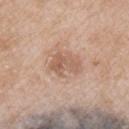Assessment: No biopsy was performed on this lesion — it was imaged during a full skin examination and was not determined to be concerning. Context: Imaged with white-light lighting. On the right upper arm. The patient is a male about 65 years old. A 15 mm close-up extracted from a 3D total-body photography capture. Longest diameter approximately 3.5 mm.